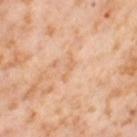The lesion was photographed on a routine skin check and not biopsied; there is no pathology result. A close-up tile cropped from a whole-body skin photograph, about 15 mm across. On the right thigh. A female patient aged 53–57. This is a cross-polarized tile.